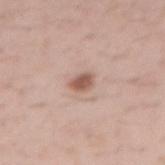This lesion was catalogued during total-body skin photography and was not selected for biopsy. Cropped from a total-body skin-imaging series; the visible field is about 15 mm. The tile uses white-light illumination. The lesion is located on the right forearm. The total-body-photography lesion software estimated a footprint of about 4 mm², an outline eccentricity of about 0.7 (0 = round, 1 = elongated), and a symmetry-axis asymmetry near 0.25. The software also gave a color-variation rating of about 2/10 and radial color variation of about 0.5. A male subject, aged 38 to 42.